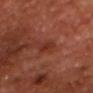No biopsy was performed on this lesion — it was imaged during a full skin examination and was not determined to be concerning.
The recorded lesion diameter is about 2.5 mm.
A male subject aged 63 to 67.
Captured under cross-polarized illumination.
This image is a 15 mm lesion crop taken from a total-body photograph.